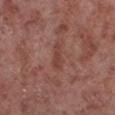Captured during whole-body skin photography for melanoma surveillance; the lesion was not biopsied.
A 15 mm crop from a total-body photograph taken for skin-cancer surveillance.
The patient is a male in their mid- to late 50s.
On the left lower leg.
The tile uses white-light illumination.